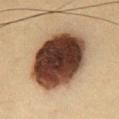Recorded during total-body skin imaging; not selected for excision or biopsy. Automated image analysis of the tile measured a footprint of about 45 mm², an eccentricity of roughly 0.55, and a shape-asymmetry score of about 0.05 (0 = symmetric). The analysis additionally found a lesion color around L≈36 a*≈17 b*≈25 in CIELAB, a lesion–skin lightness drop of about 25, and a normalized lesion–skin contrast near 19. The analysis additionally found a border-irregularity rating of about 1/10, internal color variation of about 8 on a 0–10 scale, and peripheral color asymmetry of about 2. The software also gave lesion-presence confidence of about 100/100. Located on the chest. Imaged with cross-polarized lighting. A male patient, about 35 years old. Measured at roughly 8 mm in maximum diameter. A roughly 15 mm field-of-view crop from a total-body skin photograph.